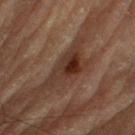follow-up: no biopsy performed (imaged during a skin exam)
lesion diameter: ≈5.5 mm
patient: male, in their mid- to late 80s or older
location: the right thigh
illumination: cross-polarized
image-analysis metrics: a mean CIELAB color near L≈26 a*≈17 b*≈22 and a lesion–skin lightness drop of about 9; border irregularity of about 6 on a 0–10 scale and peripheral color asymmetry of about 2.5
image: 15 mm crop, total-body photography